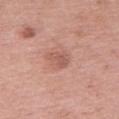| key | value |
|---|---|
| body site | the right upper arm |
| lesion diameter | ~3 mm (longest diameter) |
| patient | female, aged approximately 45 |
| acquisition | ~15 mm tile from a whole-body skin photo |
| illumination | white-light |